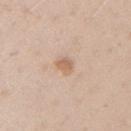Case summary:
• follow-up · total-body-photography surveillance lesion; no biopsy
• acquisition · ~15 mm tile from a whole-body skin photo
• subject · female, aged 38 to 42
• body site · the right upper arm